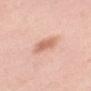Case summary:
* image: total-body-photography crop, ~15 mm field of view
* site: the mid back
* illumination: white-light illumination
* automated metrics: an average lesion color of about L≈67 a*≈23 b*≈31 (CIELAB), roughly 11 lightness units darker than nearby skin, and a normalized border contrast of about 7; a border-irregularity rating of about 2.5/10 and a peripheral color-asymmetry measure near 0.5; a nevus-likeness score of about 95/100 and lesion-presence confidence of about 100/100
* patient: female, aged approximately 40